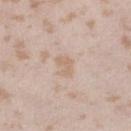On the right lower leg. Captured under white-light illumination. A 15 mm close-up extracted from a 3D total-body photography capture. A female subject aged approximately 25. The total-body-photography lesion software estimated a footprint of about 5 mm², an outline eccentricity of about 0.75 (0 = round, 1 = elongated), and a symmetry-axis asymmetry near 0.3. The software also gave a border-irregularity rating of about 3/10, internal color variation of about 1 on a 0–10 scale, and radial color variation of about 0.5. The analysis additionally found a nevus-likeness score of about 0/100 and a detector confidence of about 100 out of 100 that the crop contains a lesion.